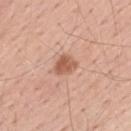Notes:
– notes · catalogued during a skin exam; not biopsied
– patient · male, in their mid-50s
– diameter · ~3 mm (longest diameter)
– image · 15 mm crop, total-body photography
– anatomic site · the mid back
– tile lighting · white-light